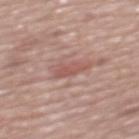{"automated_metrics": {"cielab_L": 54, "cielab_a": 23, "cielab_b": 25, "vs_skin_darker_L": 8.0, "vs_skin_contrast_norm": 5.5, "border_irregularity_0_10": 3.5, "peripheral_color_asymmetry": 0.5, "nevus_likeness_0_100": 0}, "image": {"source": "total-body photography crop", "field_of_view_mm": 15}, "site": "mid back", "lesion_size": {"long_diameter_mm_approx": 3.5}, "patient": {"sex": "male", "age_approx": 75}, "lighting": "white-light"}